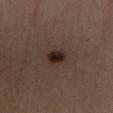Assessment:
Captured during whole-body skin photography for melanoma surveillance; the lesion was not biopsied.
Acquisition and patient details:
A 15 mm crop from a total-body photograph taken for skin-cancer surveillance. Approximately 2.5 mm at its widest. This is a cross-polarized tile. A male patient roughly 50 years of age. From the right lower leg. The total-body-photography lesion software estimated a footprint of about 4.5 mm², a shape eccentricity near 0.5, and a symmetry-axis asymmetry near 0.2. The analysis additionally found a nevus-likeness score of about 95/100 and a lesion-detection confidence of about 100/100.